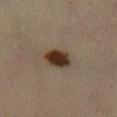location = the left lower leg; diameter = about 3.5 mm; tile lighting = cross-polarized illumination; patient = male, in their mid- to late 30s; acquisition = ~15 mm crop, total-body skin-cancer survey.